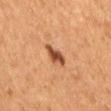Context:
A region of skin cropped from a whole-body photographic capture, roughly 15 mm wide. Located on the lower back. Longest diameter approximately 3 mm. A female subject, aged 48–52.